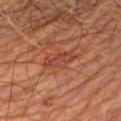An algorithmic analysis of the crop reported a footprint of about 7 mm² and two-axis asymmetry of about 0.3. The analysis additionally found a mean CIELAB color near L≈42 a*≈29 b*≈31, roughly 7 lightness units darker than nearby skin, and a normalized border contrast of about 5.5. And it measured border irregularity of about 4.5 on a 0–10 scale, a within-lesion color-variation index near 2.5/10, and radial color variation of about 1. The analysis additionally found a nevus-likeness score of about 0/100 and a detector confidence of about 100 out of 100 that the crop contains a lesion.
Imaged with cross-polarized lighting.
The recorded lesion diameter is about 4.5 mm.
On the arm.
Cropped from a whole-body photographic skin survey; the tile spans about 15 mm.
A male subject, in their mid- to late 60s.